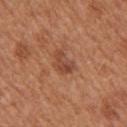No biopsy was performed on this lesion — it was imaged during a full skin examination and was not determined to be concerning. A 15 mm close-up extracted from a 3D total-body photography capture. The subject is a female aged 38 to 42. An algorithmic analysis of the crop reported an area of roughly 5 mm². The software also gave a border-irregularity rating of about 4/10, a within-lesion color-variation index near 4/10, and radial color variation of about 1.5. The lesion is on the right upper arm. The tile uses white-light illumination. About 3 mm across.